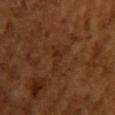The lesion was tiled from a total-body skin photograph and was not biopsied. A close-up tile cropped from a whole-body skin photograph, about 15 mm across. The lesion is on the right upper arm. A female subject aged around 55. The tile uses cross-polarized illumination.